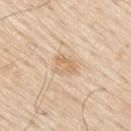Context:
A male subject, about 80 years old. The recorded lesion diameter is about 3 mm. Captured under white-light illumination. The lesion is on the right upper arm. A region of skin cropped from a whole-body photographic capture, roughly 15 mm wide. The total-body-photography lesion software estimated border irregularity of about 3 on a 0–10 scale and peripheral color asymmetry of about 1. It also reported an automated nevus-likeness rating near 10 out of 100 and a detector confidence of about 100 out of 100 that the crop contains a lesion.